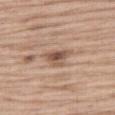Background: A close-up tile cropped from a whole-body skin photograph, about 15 mm across. Imaged with white-light lighting. On the right thigh. A male patient roughly 70 years of age. Approximately 3.5 mm at its widest.